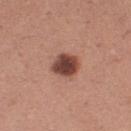workup = imaged on a skin check; not biopsied | lighting = white-light | location = the right thigh | lesion diameter = ~3 mm (longest diameter) | patient = female, approximately 30 years of age | imaging modality = ~15 mm tile from a whole-body skin photo.